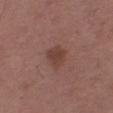{
  "biopsy_status": "not biopsied; imaged during a skin examination",
  "image": {
    "source": "total-body photography crop",
    "field_of_view_mm": 15
  },
  "site": "right thigh",
  "lesion_size": {
    "long_diameter_mm_approx": 2.5
  },
  "automated_metrics": {
    "area_mm2_approx": 5.0,
    "eccentricity": 0.45,
    "shape_asymmetry": 0.25,
    "color_variation_0_10": 2.0
  },
  "lighting": "white-light",
  "patient": {
    "sex": "male",
    "age_approx": 50
  }
}